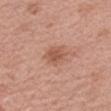  biopsy_status: not biopsied; imaged during a skin examination
  site: arm
  image:
    source: total-body photography crop
    field_of_view_mm: 15
  patient:
    sex: female
    age_approx: 65
  lesion_size:
    long_diameter_mm_approx: 3.0
  automated_metrics:
    border_irregularity_0_10: 1.5
    color_variation_0_10: 2.5
    peripheral_color_asymmetry: 1.0